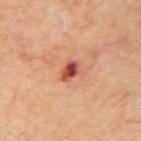notes: imaged on a skin check; not biopsied
patient: male, aged 68–72
body site: the right upper arm
diameter: about 2.5 mm
image source: ~15 mm tile from a whole-body skin photo
lighting: cross-polarized illumination
automated metrics: a border-irregularity index near 1.5/10, internal color variation of about 6.5 on a 0–10 scale, and a peripheral color-asymmetry measure near 2; a classifier nevus-likeness of about 0/100 and a lesion-detection confidence of about 100/100